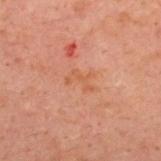No biopsy was performed on this lesion — it was imaged during a full skin examination and was not determined to be concerning. Captured under cross-polarized illumination. Cropped from a total-body skin-imaging series; the visible field is about 15 mm. The lesion is located on the upper back. A male patient aged around 40. Approximately 3 mm at its widest.